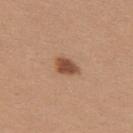<case>
<biopsy_status>not biopsied; imaged during a skin examination</biopsy_status>
<site>back</site>
<patient>
  <sex>female</sex>
  <age_approx>20</age_approx>
</patient>
<lighting>white-light</lighting>
<image>
  <source>total-body photography crop</source>
  <field_of_view_mm>15</field_of_view_mm>
</image>
<lesion_size>
  <long_diameter_mm_approx>3.0</long_diameter_mm_approx>
</lesion_size>
</case>